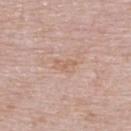Q: Is there a histopathology result?
A: no biopsy performed (imaged during a skin exam)
Q: What lighting was used for the tile?
A: white-light
Q: What kind of image is this?
A: 15 mm crop, total-body photography
Q: How large is the lesion?
A: ~3 mm (longest diameter)
Q: Patient demographics?
A: female, roughly 65 years of age
Q: What is the anatomic site?
A: the upper back